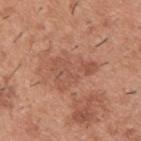The lesion was tiled from a total-body skin photograph and was not biopsied. The tile uses white-light illumination. The lesion's longest dimension is about 5 mm. A male patient about 40 years old. The lesion is on the upper back. Cropped from a total-body skin-imaging series; the visible field is about 15 mm.